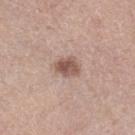Captured during whole-body skin photography for melanoma surveillance; the lesion was not biopsied. A female patient aged around 50. The lesion-visualizer software estimated a lesion area of about 5.5 mm², a shape eccentricity near 0.7, and a symmetry-axis asymmetry near 0.2. It also reported a mean CIELAB color near L≈53 a*≈19 b*≈24 and roughly 13 lightness units darker than nearby skin. The software also gave a nevus-likeness score of about 85/100 and a lesion-detection confidence of about 100/100. The tile uses white-light illumination. A 15 mm close-up extracted from a 3D total-body photography capture. The recorded lesion diameter is about 3 mm. From the right lower leg.